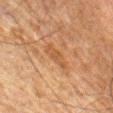The total-body-photography lesion software estimated a footprint of about 5 mm², an eccentricity of roughly 0.95, and a symmetry-axis asymmetry near 0.25. The software also gave border irregularity of about 3 on a 0–10 scale, internal color variation of about 1.5 on a 0–10 scale, and peripheral color asymmetry of about 0.5. The subject is a male roughly 80 years of age. From the abdomen. This image is a 15 mm lesion crop taken from a total-body photograph. Measured at roughly 4 mm in maximum diameter.